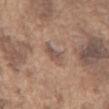Findings:
• biopsy status · no biopsy performed (imaged during a skin exam)
• acquisition · total-body-photography crop, ~15 mm field of view
• image-analysis metrics · an average lesion color of about L≈52 a*≈17 b*≈25 (CIELAB); a classifier nevus-likeness of about 0/100
• body site · the chest
• lesion size · ≈3 mm
• patient · male, aged approximately 75
• lighting · white-light